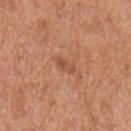Impression:
The lesion was photographed on a routine skin check and not biopsied; there is no pathology result.
Image and clinical context:
A male patient approximately 65 years of age. This image is a 15 mm lesion crop taken from a total-body photograph. The lesion's longest dimension is about 2.5 mm. Located on the right upper arm. The total-body-photography lesion software estimated a shape eccentricity near 0.9. And it measured border irregularity of about 3 on a 0–10 scale, internal color variation of about 0 on a 0–10 scale, and a peripheral color-asymmetry measure near 0. The analysis additionally found an automated nevus-likeness rating near 0 out of 100 and a lesion-detection confidence of about 100/100. Captured under white-light illumination.